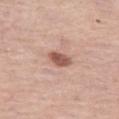follow-up: catalogued during a skin exam; not biopsied | lighting: white-light illumination | size: ~3 mm (longest diameter) | image-analysis metrics: a lesion area of about 4.5 mm² and two-axis asymmetry of about 0.25; a lesion color around L≈55 a*≈23 b*≈27 in CIELAB, roughly 14 lightness units darker than nearby skin, and a lesion-to-skin contrast of about 9.5 (normalized; higher = more distinct); a border-irregularity rating of about 2/10, a within-lesion color-variation index near 3/10, and a peripheral color-asymmetry measure near 1; an automated nevus-likeness rating near 90 out of 100 and a lesion-detection confidence of about 100/100 | location: the left thigh | imaging modality: ~15 mm crop, total-body skin-cancer survey | patient: female, about 60 years old.